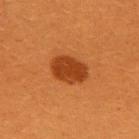Q: Was this lesion biopsied?
A: catalogued during a skin exam; not biopsied
Q: Where on the body is the lesion?
A: the upper back
Q: What is the imaging modality?
A: total-body-photography crop, ~15 mm field of view
Q: Patient demographics?
A: female, in their 30s
Q: Lesion size?
A: ~4.5 mm (longest diameter)